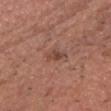Findings:
- workup — no biopsy performed (imaged during a skin exam)
- lighting — white-light illumination
- subject — male, aged approximately 75
- image-analysis metrics — a border-irregularity index near 3.5/10 and radial color variation of about 0.5; an automated nevus-likeness rating near 10 out of 100
- size — about 3 mm
- image — total-body-photography crop, ~15 mm field of view
- site — the head or neck